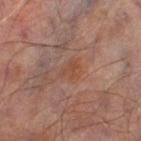The lesion was tiled from a total-body skin photograph and was not biopsied. The lesion's longest dimension is about 3 mm. The lesion is on the left thigh. A male subject, approximately 65 years of age. The tile uses cross-polarized illumination. Automated image analysis of the tile measured a mean CIELAB color near L≈45 a*≈21 b*≈29 and a normalized lesion–skin contrast near 5.5. A close-up tile cropped from a whole-body skin photograph, about 15 mm across.